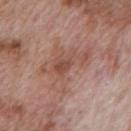Imaged during a routine full-body skin examination; the lesion was not biopsied and no histopathology is available.
A male patient, aged around 70.
This image is a 15 mm lesion crop taken from a total-body photograph.
This is a white-light tile.
The lesion is located on the mid back.